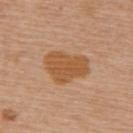Part of a total-body skin-imaging series; this lesion was reviewed on a skin check and was not flagged for biopsy.